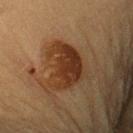Impression: The lesion was tiled from a total-body skin photograph and was not biopsied. Acquisition and patient details: This image is a 15 mm lesion crop taken from a total-body photograph. A female subject aged 58–62. About 5.5 mm across. The lesion is on the right upper arm.